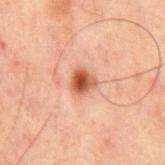This lesion was catalogued during total-body skin photography and was not selected for biopsy. The tile uses cross-polarized illumination. Cropped from a total-body skin-imaging series; the visible field is about 15 mm. Located on the mid back. About 2.5 mm across. A male patient, aged around 60. The lesion-visualizer software estimated roughly 13 lightness units darker than nearby skin. And it measured border irregularity of about 2.5 on a 0–10 scale, a within-lesion color-variation index near 7/10, and peripheral color asymmetry of about 2.